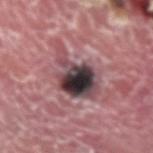A lesion tile, about 15 mm wide, cut from a 3D total-body photograph.
Captured under white-light illumination.
The lesion is on the right upper arm.
The lesion-visualizer software estimated a color-variation rating of about 10/10 and a peripheral color-asymmetry measure near 6. And it measured a classifier nevus-likeness of about 0/100.
The patient is a male in their mid- to late 60s.